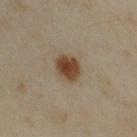Findings:
* body site — the arm
* imaging modality — 15 mm crop, total-body photography
* lesion diameter — about 4 mm
* subject — female, in their mid-30s
* tile lighting — cross-polarized illumination